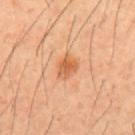Case summary:
- notes — catalogued during a skin exam; not biopsied
- illumination — cross-polarized illumination
- image — ~15 mm crop, total-body skin-cancer survey
- location — the mid back
- patient — male, roughly 40 years of age